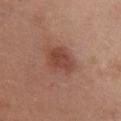Clinical impression: This lesion was catalogued during total-body skin photography and was not selected for biopsy. Clinical summary: Imaged with white-light lighting. The lesion is located on the chest. The lesion-visualizer software estimated a lesion area of about 10 mm², an outline eccentricity of about 0.7 (0 = round, 1 = elongated), and a shape-asymmetry score of about 0.2 (0 = symmetric). The analysis additionally found roughly 9 lightness units darker than nearby skin and a normalized lesion–skin contrast near 7. A female subject, about 50 years old. A 15 mm close-up tile from a total-body photography series done for melanoma screening.